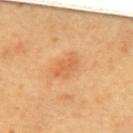Q: Automated lesion metrics?
A: two-axis asymmetry of about 0.25; a mean CIELAB color near L≈65 a*≈26 b*≈44 and a normalized border contrast of about 5; a within-lesion color-variation index near 2/10 and peripheral color asymmetry of about 0.5; a classifier nevus-likeness of about 5/100 and a lesion-detection confidence of about 100/100
Q: What are the patient's age and sex?
A: female, aged 58–62
Q: What is the lesion's diameter?
A: about 3.5 mm
Q: What is the anatomic site?
A: the upper back
Q: How was this image acquired?
A: ~15 mm crop, total-body skin-cancer survey